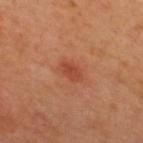<case>
  <lighting>cross-polarized</lighting>
  <site>upper back</site>
  <lesion_size>
    <long_diameter_mm_approx>3.0</long_diameter_mm_approx>
  </lesion_size>
  <patient>
    <sex>male</sex>
    <age_approx>50</age_approx>
  </patient>
  <image>
    <source>total-body photography crop</source>
    <field_of_view_mm>15</field_of_view_mm>
  </image>
</case>